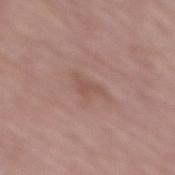Notes:
• biopsy status — catalogued during a skin exam; not biopsied